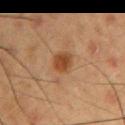Q: Was a biopsy performed?
A: imaged on a skin check; not biopsied
Q: What kind of image is this?
A: 15 mm crop, total-body photography
Q: What did automated image analysis measure?
A: an area of roughly 5.5 mm², a shape eccentricity near 0.45, and a shape-asymmetry score of about 0.2 (0 = symmetric); about 9 CIELAB-L* units darker than the surrounding skin and a normalized lesion–skin contrast near 8.5; a border-irregularity index near 1.5/10 and a within-lesion color-variation index near 2/10; a nevus-likeness score of about 90/100 and a detector confidence of about 100 out of 100 that the crop contains a lesion
Q: Illumination type?
A: cross-polarized illumination
Q: Where on the body is the lesion?
A: the right upper arm
Q: Who is the patient?
A: male, about 55 years old